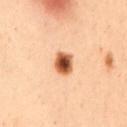- workup — total-body-photography surveillance lesion; no biopsy
- patient — female, roughly 50 years of age
- size — ≈3 mm
- imaging modality — ~15 mm crop, total-body skin-cancer survey
- site — the back